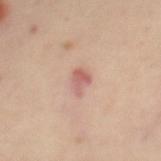Impression:
This lesion was catalogued during total-body skin photography and was not selected for biopsy.
Image and clinical context:
Automated image analysis of the tile measured an area of roughly 3.5 mm², a shape eccentricity near 0.7, and a symmetry-axis asymmetry near 0.35. The software also gave a nevus-likeness score of about 10/100 and a detector confidence of about 100 out of 100 that the crop contains a lesion. Captured under cross-polarized illumination. A female subject, aged 43–47. The lesion is on the abdomen. Approximately 2.5 mm at its widest. A roughly 15 mm field-of-view crop from a total-body skin photograph.